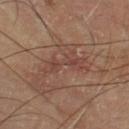Impression:
Recorded during total-body skin imaging; not selected for excision or biopsy.
Background:
Cropped from a total-body skin-imaging series; the visible field is about 15 mm. Located on the right lower leg. A male patient aged approximately 65.